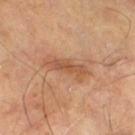follow-up: imaged on a skin check; not biopsied
acquisition: ~15 mm tile from a whole-body skin photo
subject: aged 63 to 67
illumination: cross-polarized
body site: the right thigh
automated lesion analysis: about 9 CIELAB-L* units darker than the surrounding skin; a within-lesion color-variation index near 5/10 and peripheral color asymmetry of about 2; a classifier nevus-likeness of about 0/100
lesion size: ~5.5 mm (longest diameter)